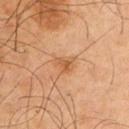- workup — imaged on a skin check; not biopsied
- anatomic site — the left upper arm
- lesion size — ~2.5 mm (longest diameter)
- TBP lesion metrics — a lesion area of about 4 mm², a shape eccentricity near 0.6, and two-axis asymmetry of about 0.35; a border-irregularity index near 3.5/10, a within-lesion color-variation index near 2.5/10, and a peripheral color-asymmetry measure near 1; an automated nevus-likeness rating near 35 out of 100 and a detector confidence of about 100 out of 100 that the crop contains a lesion
- patient — male, in their mid-60s
- image — ~15 mm tile from a whole-body skin photo
- illumination — cross-polarized illumination